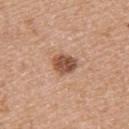Q: Was a biopsy performed?
A: imaged on a skin check; not biopsied
Q: What kind of image is this?
A: 15 mm crop, total-body photography
Q: What lighting was used for the tile?
A: white-light illumination
Q: Where on the body is the lesion?
A: the left upper arm
Q: Who is the patient?
A: female, aged 68–72